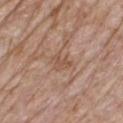Assessment: The lesion was photographed on a routine skin check and not biopsied; there is no pathology result. Background: The lesion is located on the chest. An algorithmic analysis of the crop reported border irregularity of about 2.5 on a 0–10 scale, internal color variation of about 3 on a 0–10 scale, and radial color variation of about 1. The software also gave a classifier nevus-likeness of about 0/100 and a lesion-detection confidence of about 75/100. The patient is a female aged 68–72. About 3.5 mm across. This image is a 15 mm lesion crop taken from a total-body photograph.